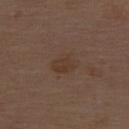Q: Was this lesion biopsied?
A: no biopsy performed (imaged during a skin exam)
Q: What is the lesion's diameter?
A: ≈3 mm
Q: Lesion location?
A: the upper back
Q: What is the imaging modality?
A: 15 mm crop, total-body photography
Q: What are the patient's age and sex?
A: male, about 70 years old
Q: Illumination type?
A: white-light illumination
Q: What did automated image analysis measure?
A: a symmetry-axis asymmetry near 0.25; a border-irregularity index near 2.5/10, a within-lesion color-variation index near 2/10, and peripheral color asymmetry of about 0.5